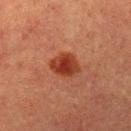Q: Was this lesion biopsied?
A: imaged on a skin check; not biopsied
Q: How was the tile lit?
A: cross-polarized
Q: Who is the patient?
A: female, about 55 years old
Q: What did automated image analysis measure?
A: a shape eccentricity near 0.65 and a shape-asymmetry score of about 0.2 (0 = symmetric); a lesion color around L≈32 a*≈27 b*≈29 in CIELAB, a lesion–skin lightness drop of about 10, and a normalized lesion–skin contrast near 10; a border-irregularity rating of about 2/10, a color-variation rating of about 4/10, and peripheral color asymmetry of about 1; a nevus-likeness score of about 100/100 and a detector confidence of about 100 out of 100 that the crop contains a lesion
Q: How was this image acquired?
A: total-body-photography crop, ~15 mm field of view
Q: What is the anatomic site?
A: the left upper arm
Q: What is the lesion's diameter?
A: ~3.5 mm (longest diameter)